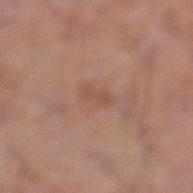{
  "biopsy_status": "not biopsied; imaged during a skin examination",
  "site": "left lower leg",
  "image": {
    "source": "total-body photography crop",
    "field_of_view_mm": 15
  },
  "lighting": "white-light",
  "lesion_size": {
    "long_diameter_mm_approx": 2.5
  },
  "patient": {
    "sex": "male",
    "age_approx": 20
  }
}